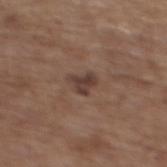Impression:
This lesion was catalogued during total-body skin photography and was not selected for biopsy.
Background:
A female patient aged approximately 65. The lesion's longest dimension is about 2.5 mm. A 15 mm close-up tile from a total-body photography series done for melanoma screening. Located on the upper back. Automated tile analysis of the lesion measured an automated nevus-likeness rating near 5 out of 100 and a detector confidence of about 100 out of 100 that the crop contains a lesion. Captured under white-light illumination.